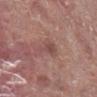Part of a total-body skin-imaging series; this lesion was reviewed on a skin check and was not flagged for biopsy. A female patient aged around 80. On the left leg. Captured under cross-polarized illumination. A 15 mm close-up tile from a total-body photography series done for melanoma screening.